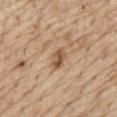This lesion was catalogued during total-body skin photography and was not selected for biopsy. From the abdomen. A 15 mm close-up extracted from a 3D total-body photography capture. Captured under white-light illumination. A male patient, about 70 years old. Measured at roughly 3 mm in maximum diameter. The lesion-visualizer software estimated a footprint of about 4.5 mm², an outline eccentricity of about 0.75 (0 = round, 1 = elongated), and a shape-asymmetry score of about 0.2 (0 = symmetric).